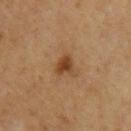Assessment: The lesion was photographed on a routine skin check and not biopsied; there is no pathology result. Acquisition and patient details: The tile uses cross-polarized illumination. The lesion is on the right upper arm. A male patient, aged around 60. The total-body-photography lesion software estimated a footprint of about 4.5 mm², an eccentricity of roughly 0.55, and a shape-asymmetry score of about 0.3 (0 = symmetric). And it measured a lesion color around L≈39 a*≈20 b*≈33 in CIELAB, about 10 CIELAB-L* units darker than the surrounding skin, and a lesion-to-skin contrast of about 8.5 (normalized; higher = more distinct). It also reported an automated nevus-likeness rating near 95 out of 100 and a lesion-detection confidence of about 100/100. Cropped from a whole-body photographic skin survey; the tile spans about 15 mm.